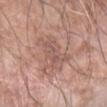Part of a total-body skin-imaging series; this lesion was reviewed on a skin check and was not flagged for biopsy. This is a white-light tile. Approximately 5 mm at its widest. Located on the right forearm. Cropped from a whole-body photographic skin survey; the tile spans about 15 mm. The patient is a male aged 58 to 62. An algorithmic analysis of the crop reported a lesion area of about 12 mm² and a shape-asymmetry score of about 0.4 (0 = symmetric). The analysis additionally found a border-irregularity index near 5.5/10, a within-lesion color-variation index near 3.5/10, and a peripheral color-asymmetry measure near 1.5.